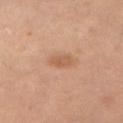Assessment:
This lesion was catalogued during total-body skin photography and was not selected for biopsy.
Image and clinical context:
Measured at roughly 2.5 mm in maximum diameter. The patient is a female aged approximately 50. Located on the left thigh. A 15 mm crop from a total-body photograph taken for skin-cancer surveillance. An algorithmic analysis of the crop reported a lesion color around L≈55 a*≈21 b*≈33 in CIELAB, a lesion–skin lightness drop of about 8, and a normalized border contrast of about 6. This is a cross-polarized tile.